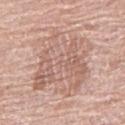Case summary:
* biopsy status: no biopsy performed (imaged during a skin exam)
* imaging modality: ~15 mm crop, total-body skin-cancer survey
* diameter: ~8.5 mm (longest diameter)
* lighting: white-light
* body site: the leg
* patient: female, aged 58–62